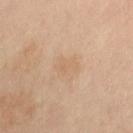Impression: The lesion was photographed on a routine skin check and not biopsied; there is no pathology result.